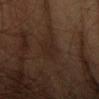biopsy_status: not biopsied; imaged during a skin examination
site: right forearm
image:
  source: total-body photography crop
  field_of_view_mm: 15
lighting: cross-polarized
patient:
  sex: male
  age_approx: 60
automated_metrics:
  eccentricity: 0.8
  shape_asymmetry: 0.2
  cielab_L: 21
  cielab_a: 13
  cielab_b: 19
  vs_skin_darker_L: 4.0
  vs_skin_contrast_norm: 5.0
  border_irregularity_0_10: 3.0
  color_variation_0_10: 2.0
  peripheral_color_asymmetry: 0.5
  nevus_likeness_0_100: 0
  lesion_detection_confidence_0_100: 85